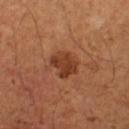Recorded during total-body skin imaging; not selected for excision or biopsy. The patient is a male approximately 65 years of age. A close-up tile cropped from a whole-body skin photograph, about 15 mm across.